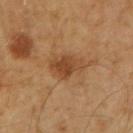Assessment: The lesion was tiled from a total-body skin photograph and was not biopsied. Clinical summary: A male subject, aged around 60. From the right upper arm. A 15 mm close-up extracted from a 3D total-body photography capture.